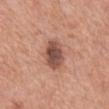Assessment:
Captured during whole-body skin photography for melanoma surveillance; the lesion was not biopsied.
Clinical summary:
Located on the mid back. About 5 mm across. A male patient aged 68 to 72. A 15 mm close-up tile from a total-body photography series done for melanoma screening.